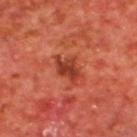Q: Illumination type?
A: cross-polarized illumination
Q: What is the lesion's diameter?
A: about 4 mm
Q: Patient demographics?
A: male, aged around 65
Q: What kind of image is this?
A: ~15 mm crop, total-body skin-cancer survey
Q: Lesion location?
A: the upper back